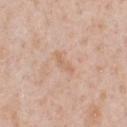The lesion was tiled from a total-body skin photograph and was not biopsied. A male subject, aged approximately 50. The lesion-visualizer software estimated a border-irregularity index near 5/10 and radial color variation of about 0. Located on the chest. This is a white-light tile. A region of skin cropped from a whole-body photographic capture, roughly 15 mm wide.